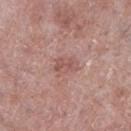notes = catalogued during a skin exam; not biopsied
illumination = white-light illumination
image-analysis metrics = an automated nevus-likeness rating near 0 out of 100 and lesion-presence confidence of about 100/100
lesion size = ≈3 mm
patient = male, in their mid-70s
body site = the right lower leg
imaging modality = ~15 mm tile from a whole-body skin photo